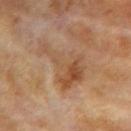Q: Was a biopsy performed?
A: total-body-photography surveillance lesion; no biopsy
Q: What kind of image is this?
A: 15 mm crop, total-body photography
Q: Who is the patient?
A: female, roughly 75 years of age
Q: How large is the lesion?
A: about 6.5 mm
Q: What is the anatomic site?
A: the left upper arm
Q: What lighting was used for the tile?
A: cross-polarized